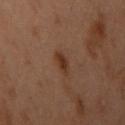follow-up = imaged on a skin check; not biopsied | tile lighting = cross-polarized illumination | imaging modality = ~15 mm crop, total-body skin-cancer survey | lesion diameter = ~2.5 mm (longest diameter) | subject = female, aged around 55 | site = the arm.